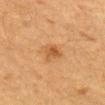  biopsy_status: not biopsied; imaged during a skin examination
  site: left upper arm
  patient:
    sex: male
    age_approx: 85+
  automated_metrics:
    area_mm2_approx: 4.5
    eccentricity: 0.7
    shape_asymmetry: 0.4
    cielab_L: 48
    cielab_a: 21
    cielab_b: 38
    nevus_likeness_0_100: 70
    lesion_detection_confidence_0_100: 100
  lighting: cross-polarized
  image:
    source: total-body photography crop
    field_of_view_mm: 15